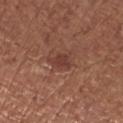Part of a total-body skin-imaging series; this lesion was reviewed on a skin check and was not flagged for biopsy.
A roughly 15 mm field-of-view crop from a total-body skin photograph.
The subject is a female roughly 65 years of age.
Imaged with white-light lighting.
Automated tile analysis of the lesion measured an area of roughly 3.5 mm² and a shape eccentricity near 0.7. It also reported a mean CIELAB color near L≈39 a*≈24 b*≈25, a lesion–skin lightness drop of about 8, and a lesion-to-skin contrast of about 6.5 (normalized; higher = more distinct). The analysis additionally found border irregularity of about 3.5 on a 0–10 scale and radial color variation of about 0.5. The analysis additionally found lesion-presence confidence of about 100/100.
On the right upper arm.
The lesion's longest dimension is about 2.5 mm.